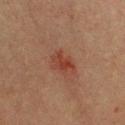Clinical summary: A male subject, in their 40s. Cropped from a total-body skin-imaging series; the visible field is about 15 mm. Located on the chest. An algorithmic analysis of the crop reported a lesion area of about 5 mm² and a shape-asymmetry score of about 0.25 (0 = symmetric). It also reported a border-irregularity index near 3.5/10 and peripheral color asymmetry of about 1.5.